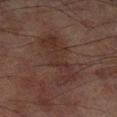notes: total-body-photography surveillance lesion; no biopsy | illumination: cross-polarized | acquisition: 15 mm crop, total-body photography | subject: male, aged 68–72 | site: the left lower leg.